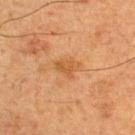Q: Was a biopsy performed?
A: catalogued during a skin exam; not biopsied
Q: How was the tile lit?
A: cross-polarized illumination
Q: How was this image acquired?
A: ~15 mm crop, total-body skin-cancer survey
Q: Patient demographics?
A: male, in their 60s
Q: What is the lesion's diameter?
A: ~3 mm (longest diameter)
Q: Where on the body is the lesion?
A: the upper back